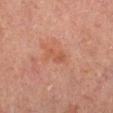workup — no biopsy performed (imaged during a skin exam); anatomic site — the leg; subject — male, approximately 65 years of age; image — ~15 mm tile from a whole-body skin photo; size — ≈3 mm; tile lighting — cross-polarized illumination.